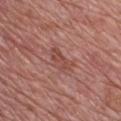Q: Is there a histopathology result?
A: catalogued during a skin exam; not biopsied
Q: How was this image acquired?
A: ~15 mm crop, total-body skin-cancer survey
Q: What is the anatomic site?
A: the front of the torso
Q: Who is the patient?
A: male, aged around 50
Q: What lighting was used for the tile?
A: white-light illumination